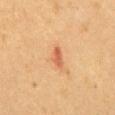Notes:
- acquisition · ~15 mm crop, total-body skin-cancer survey
- subject · male, aged approximately 55
- tile lighting · cross-polarized illumination
- diameter · about 3 mm
- body site · the chest